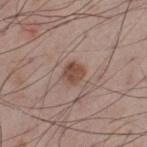Assessment: Imaged during a routine full-body skin examination; the lesion was not biopsied and no histopathology is available. Context: The tile uses white-light illumination. A male patient, roughly 60 years of age. The total-body-photography lesion software estimated a lesion color around L≈48 a*≈19 b*≈25 in CIELAB, a lesion–skin lightness drop of about 10, and a lesion-to-skin contrast of about 8 (normalized; higher = more distinct). The analysis additionally found border irregularity of about 1.5 on a 0–10 scale and peripheral color asymmetry of about 1. The software also gave a classifier nevus-likeness of about 90/100. Longest diameter approximately 2.5 mm. Cropped from a total-body skin-imaging series; the visible field is about 15 mm. Located on the leg.